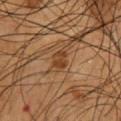Impression:
Captured during whole-body skin photography for melanoma surveillance; the lesion was not biopsied.
Clinical summary:
A lesion tile, about 15 mm wide, cut from a 3D total-body photograph. A male subject, aged around 55. Automated tile analysis of the lesion measured a footprint of about 3.5 mm², an eccentricity of roughly 0.85, and a symmetry-axis asymmetry near 0.25. And it measured a border-irregularity rating of about 3.5/10, a within-lesion color-variation index near 1/10, and a peripheral color-asymmetry measure near 0.5. The recorded lesion diameter is about 2.5 mm. Located on the chest. Imaged with cross-polarized lighting.